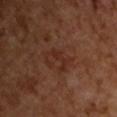Recorded during total-body skin imaging; not selected for excision or biopsy.
Cropped from a total-body skin-imaging series; the visible field is about 15 mm.
The lesion-visualizer software estimated a lesion area of about 4 mm², an eccentricity of roughly 0.9, and a symmetry-axis asymmetry near 0.55. The analysis additionally found a border-irregularity index near 7.5/10, internal color variation of about 0 on a 0–10 scale, and a peripheral color-asymmetry measure near 0.
Captured under cross-polarized illumination.
About 4 mm across.
A male patient about 65 years old.